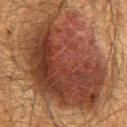The lesion was tiled from a total-body skin photograph and was not biopsied.
The patient is a male approximately 60 years of age.
The lesion-visualizer software estimated a lesion color around L≈31 a*≈19 b*≈24 in CIELAB and a lesion-to-skin contrast of about 11.5 (normalized; higher = more distinct). And it measured a border-irregularity rating of about 4.5/10, a within-lesion color-variation index near 6.5/10, and peripheral color asymmetry of about 2. The software also gave a nevus-likeness score of about 75/100 and lesion-presence confidence of about 100/100.
Approximately 13.5 mm at its widest.
Captured under cross-polarized illumination.
Located on the mid back.
A close-up tile cropped from a whole-body skin photograph, about 15 mm across.